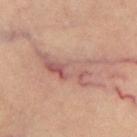image source: total-body-photography crop, ~15 mm field of view | anatomic site: the left thigh | lesion size: ≈7 mm | tile lighting: cross-polarized illumination | TBP lesion metrics: an area of roughly 15 mm² and an outline eccentricity of about 0.9 (0 = round, 1 = elongated); border irregularity of about 6.5 on a 0–10 scale and radial color variation of about 2.5 | subject: female, aged 63 to 67.